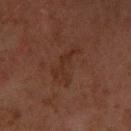Acquisition and patient details: A 15 mm close-up tile from a total-body photography series done for melanoma screening. Automated tile analysis of the lesion measured a lesion color around L≈26 a*≈18 b*≈23 in CIELAB and a normalized border contrast of about 5. And it measured an automated nevus-likeness rating near 5 out of 100. The patient is a female aged around 60. The recorded lesion diameter is about 4.5 mm. The lesion is located on the right upper arm. Captured under cross-polarized illumination.